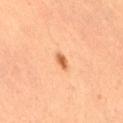Assessment:
The lesion was tiled from a total-body skin photograph and was not biopsied.
Background:
A female subject roughly 35 years of age. The lesion is located on the right thigh. A 15 mm close-up extracted from a 3D total-body photography capture.